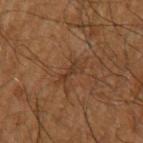Impression:
No biopsy was performed on this lesion — it was imaged during a full skin examination and was not determined to be concerning.
Image and clinical context:
A 15 mm crop from a total-body photograph taken for skin-cancer surveillance. The total-body-photography lesion software estimated a shape eccentricity near 0.9 and two-axis asymmetry of about 0.35. And it measured border irregularity of about 5.5 on a 0–10 scale, a within-lesion color-variation index near 0/10, and radial color variation of about 0. Longest diameter approximately 3 mm. The tile uses cross-polarized illumination. A male subject, approximately 60 years of age. Located on the left upper arm.